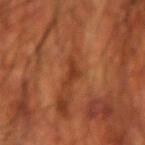Captured during whole-body skin photography for melanoma surveillance; the lesion was not biopsied. Measured at roughly 3 mm in maximum diameter. A male subject about 70 years old. This image is a 15 mm lesion crop taken from a total-body photograph. The lesion is on the left forearm.